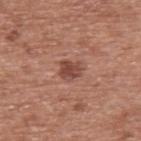Clinical impression:
Captured during whole-body skin photography for melanoma surveillance; the lesion was not biopsied.
Background:
A male patient, aged 73 to 77. Cropped from a whole-body photographic skin survey; the tile spans about 15 mm. The lesion is located on the upper back.